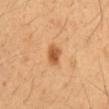Recorded during total-body skin imaging; not selected for excision or biopsy. Automated image analysis of the tile measured an area of roughly 4.5 mm² and a shape eccentricity near 0.65. The analysis additionally found an average lesion color of about L≈56 a*≈25 b*≈41 (CIELAB), roughly 13 lightness units darker than nearby skin, and a normalized lesion–skin contrast near 8.5. It also reported border irregularity of about 1.5 on a 0–10 scale and a within-lesion color-variation index near 3/10. Located on the abdomen. Captured under cross-polarized illumination. A male subject aged around 50. Cropped from a total-body skin-imaging series; the visible field is about 15 mm.